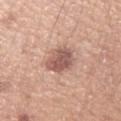notes = no biopsy performed (imaged during a skin exam)
image = total-body-photography crop, ~15 mm field of view
body site = the arm
patient = female, roughly 45 years of age